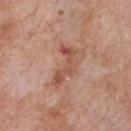Case summary:
– workup — no biopsy performed (imaged during a skin exam)
– size — ~5 mm (longest diameter)
– image-analysis metrics — a footprint of about 7 mm² and an outline eccentricity of about 0.9 (0 = round, 1 = elongated); a classifier nevus-likeness of about 0/100 and a lesion-detection confidence of about 100/100
– subject — male, about 60 years old
– tile lighting — white-light illumination
– site — the chest
– image — total-body-photography crop, ~15 mm field of view